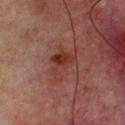Findings:
* workup — total-body-photography surveillance lesion; no biopsy
* diameter — about 4 mm
* image source — 15 mm crop, total-body photography
* patient — male, aged 63 to 67
* anatomic site — the back